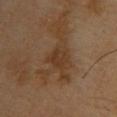Clinical impression: Captured during whole-body skin photography for melanoma surveillance; the lesion was not biopsied.